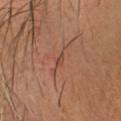Q: Where on the body is the lesion?
A: the head or neck
Q: What kind of image is this?
A: ~15 mm crop, total-body skin-cancer survey
Q: Patient demographics?
A: male, aged 63–67